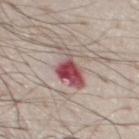Assessment:
The lesion was photographed on a routine skin check and not biopsied; there is no pathology result.
Image and clinical context:
The patient is a male about 80 years old. The lesion is located on the chest. A lesion tile, about 15 mm wide, cut from a 3D total-body photograph. Measured at roughly 6 mm in maximum diameter.